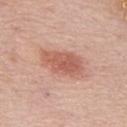The lesion was tiled from a total-body skin photograph and was not biopsied. A region of skin cropped from a whole-body photographic capture, roughly 15 mm wide. The recorded lesion diameter is about 5.5 mm. Automated image analysis of the tile measured a lesion area of about 13 mm² and an eccentricity of roughly 0.85. The analysis additionally found roughly 11 lightness units darker than nearby skin. The patient is a male aged 73 to 77. Imaged with white-light lighting. From the back.